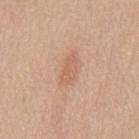notes: imaged on a skin check; not biopsied
subject: male, in their 50s
image: ~15 mm crop, total-body skin-cancer survey
body site: the mid back